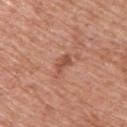<tbp_lesion>
  <automated_metrics>
    <area_mm2_approx>3.0</area_mm2_approx>
    <shape_asymmetry>0.35</shape_asymmetry>
    <cielab_L>51</cielab_L>
    <cielab_a>25</cielab_a>
    <cielab_b>31</cielab_b>
    <vs_skin_darker_L>10.0</vs_skin_darker_L>
    <vs_skin_contrast_norm>7.0</vs_skin_contrast_norm>
    <lesion_detection_confidence_0_100>100</lesion_detection_confidence_0_100>
  </automated_metrics>
  <patient>
    <sex>male</sex>
    <age_approx>60</age_approx>
  </patient>
  <lighting>white-light</lighting>
  <lesion_size>
    <long_diameter_mm_approx>2.5</long_diameter_mm_approx>
  </lesion_size>
  <site>upper back</site>
  <image>
    <source>total-body photography crop</source>
    <field_of_view_mm>15</field_of_view_mm>
  </image>
</tbp_lesion>